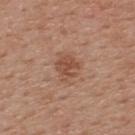{"biopsy_status": "not biopsied; imaged during a skin examination", "lighting": "white-light", "patient": {"sex": "male", "age_approx": 55}, "site": "upper back", "lesion_size": {"long_diameter_mm_approx": 2.5}, "image": {"source": "total-body photography crop", "field_of_view_mm": 15}}